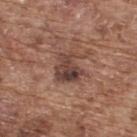Captured during whole-body skin photography for melanoma surveillance; the lesion was not biopsied.
A male patient, roughly 75 years of age.
On the upper back.
This is a white-light tile.
The lesion's longest dimension is about 3.5 mm.
This image is a 15 mm lesion crop taken from a total-body photograph.